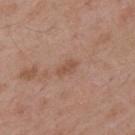Clinical impression: Imaged during a routine full-body skin examination; the lesion was not biopsied and no histopathology is available. Image and clinical context: Approximately 3 mm at its widest. The lesion-visualizer software estimated border irregularity of about 2.5 on a 0–10 scale, internal color variation of about 1 on a 0–10 scale, and radial color variation of about 0.5. The software also gave an automated nevus-likeness rating near 0 out of 100 and lesion-presence confidence of about 100/100. Cropped from a whole-body photographic skin survey; the tile spans about 15 mm. On the mid back. A male patient, aged around 55. Imaged with white-light lighting.